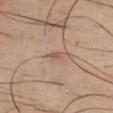notes: imaged on a skin check; not biopsied | site: the chest | imaging modality: 15 mm crop, total-body photography | tile lighting: cross-polarized illumination | subject: male, aged 38–42 | TBP lesion metrics: an area of roughly 2.5 mm² and a shape-asymmetry score of about 0.35 (0 = symmetric); about 7 CIELAB-L* units darker than the surrounding skin and a lesion-to-skin contrast of about 5 (normalized; higher = more distinct); internal color variation of about 0.5 on a 0–10 scale and radial color variation of about 0.